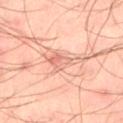{"biopsy_status": "not biopsied; imaged during a skin examination", "lighting": "cross-polarized", "image": {"source": "total-body photography crop", "field_of_view_mm": 15}, "site": "left thigh", "lesion_size": {"long_diameter_mm_approx": 4.0}, "patient": {"sex": "male", "age_approx": 45}}